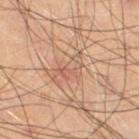Imaged during a routine full-body skin examination; the lesion was not biopsied and no histopathology is available. The lesion is on the right thigh. The lesion-visualizer software estimated an average lesion color of about L≈58 a*≈20 b*≈29 (CIELAB), a lesion–skin lightness drop of about 7, and a normalized lesion–skin contrast near 5. A lesion tile, about 15 mm wide, cut from a 3D total-body photograph. The tile uses cross-polarized illumination. A male subject aged around 70.